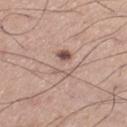<lesion>
  <biopsy_status>not biopsied; imaged during a skin examination</biopsy_status>
  <image>
    <source>total-body photography crop</source>
    <field_of_view_mm>15</field_of_view_mm>
  </image>
  <site>left thigh</site>
  <lesion_size>
    <long_diameter_mm_approx>4.5</long_diameter_mm_approx>
  </lesion_size>
  <patient>
    <sex>male</sex>
    <age_approx>55</age_approx>
  </patient>
</lesion>